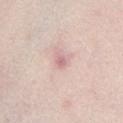Captured during whole-body skin photography for melanoma surveillance; the lesion was not biopsied.
The tile uses white-light illumination.
Approximately 1.5 mm at its widest.
Located on the chest.
Cropped from a whole-body photographic skin survey; the tile spans about 15 mm.
A female patient about 65 years old.